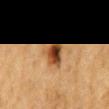Automated tile analysis of the lesion measured an average lesion color of about L≈40 a*≈20 b*≈34 (CIELAB) and a normalized lesion–skin contrast near 12. It also reported a border-irregularity index near 3/10, a within-lesion color-variation index near 10/10, and a peripheral color-asymmetry measure near 4. It also reported an automated nevus-likeness rating near 100 out of 100 and a detector confidence of about 100 out of 100 that the crop contains a lesion. A male patient roughly 85 years of age or older. Located on the mid back. The lesion's longest dimension is about 3.5 mm. A 15 mm close-up tile from a total-body photography series done for melanoma screening. The tile uses cross-polarized illumination.